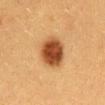| key | value |
|---|---|
| notes | catalogued during a skin exam; not biopsied |
| location | the front of the torso |
| image | ~15 mm tile from a whole-body skin photo |
| lesion size | ~4 mm (longest diameter) |
| subject | female, aged 38–42 |
| illumination | cross-polarized illumination |